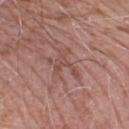• biopsy status — no biopsy performed (imaged during a skin exam)
• site — the left lower leg
• image source — 15 mm crop, total-body photography
• lesion size — about 4 mm
• automated metrics — a border-irregularity index near 9.5/10 and a color-variation rating of about 1.5/10
• illumination — white-light
• subject — male, aged 73 to 77